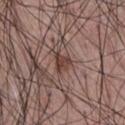| key | value |
|---|---|
| follow-up | no biopsy performed (imaged during a skin exam) |